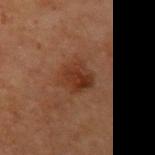Q: Is there a histopathology result?
A: catalogued during a skin exam; not biopsied
Q: What lighting was used for the tile?
A: cross-polarized illumination
Q: How large is the lesion?
A: ≈3.5 mm
Q: What is the anatomic site?
A: the arm
Q: Who is the patient?
A: male, aged 68 to 72
Q: What kind of image is this?
A: ~15 mm crop, total-body skin-cancer survey